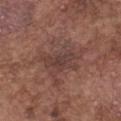| feature | finding |
|---|---|
| notes | no biopsy performed (imaged during a skin exam) |
| image | ~15 mm crop, total-body skin-cancer survey |
| diameter | ~6.5 mm (longest diameter) |
| lighting | white-light |
| patient | male, aged 73 to 77 |
| anatomic site | the chest |
| automated metrics | a footprint of about 21 mm², an outline eccentricity of about 0.5 (0 = round, 1 = elongated), and a shape-asymmetry score of about 0.45 (0 = symmetric); roughly 7 lightness units darker than nearby skin and a lesion-to-skin contrast of about 6 (normalized; higher = more distinct); an automated nevus-likeness rating near 0 out of 100 and a lesion-detection confidence of about 95/100 |